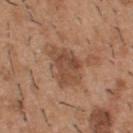Imaged during a routine full-body skin examination; the lesion was not biopsied and no histopathology is available.
A male patient, roughly 40 years of age.
Imaged with white-light lighting.
About 4.5 mm across.
Located on the back.
Cropped from a whole-body photographic skin survey; the tile spans about 15 mm.